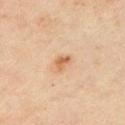Clinical summary: About 2.5 mm across. A male subject, about 45 years old. A 15 mm crop from a total-body photograph taken for skin-cancer surveillance. Located on the left upper arm. Imaged with cross-polarized lighting.